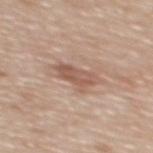Impression:
This lesion was catalogued during total-body skin photography and was not selected for biopsy.
Acquisition and patient details:
The tile uses white-light illumination. About 4 mm across. On the upper back. The lesion-visualizer software estimated a border-irregularity index near 5/10, a color-variation rating of about 3.5/10, and a peripheral color-asymmetry measure near 1.5. A male patient, aged 68–72. Cropped from a total-body skin-imaging series; the visible field is about 15 mm.